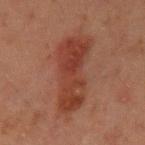Recorded during total-body skin imaging; not selected for excision or biopsy. Measured at roughly 8 mm in maximum diameter. A male patient, aged around 45. A 15 mm close-up tile from a total-body photography series done for melanoma screening. The tile uses cross-polarized illumination. From the left upper arm.